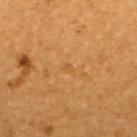  biopsy_status: not biopsied; imaged during a skin examination
  patient:
    sex: male
    age_approx: 50
  site: upper back
  image:
    source: total-body photography crop
    field_of_view_mm: 15